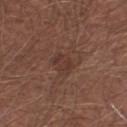Clinical impression: This lesion was catalogued during total-body skin photography and was not selected for biopsy. Image and clinical context: Longest diameter approximately 2.5 mm. The lesion is on the right lower leg. Captured under white-light illumination. The patient is a male roughly 65 years of age. Automated image analysis of the tile measured an area of roughly 5 mm², an outline eccentricity of about 0.5 (0 = round, 1 = elongated), and two-axis asymmetry of about 0.25. It also reported an automated nevus-likeness rating near 25 out of 100 and a lesion-detection confidence of about 100/100. A 15 mm close-up extracted from a 3D total-body photography capture.